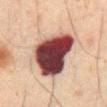Clinical impression:
This lesion was catalogued during total-body skin photography and was not selected for biopsy.
Clinical summary:
From the mid back. A male patient roughly 70 years of age. The recorded lesion diameter is about 7 mm. Cropped from a total-body skin-imaging series; the visible field is about 15 mm.